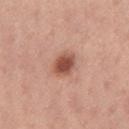The lesion was tiled from a total-body skin photograph and was not biopsied. Longest diameter approximately 3.5 mm. A 15 mm close-up extracted from a 3D total-body photography capture. From the leg. A female patient, about 25 years old. The lesion-visualizer software estimated a footprint of about 7 mm² and a shape eccentricity near 0.65. The analysis additionally found internal color variation of about 4 on a 0–10 scale and radial color variation of about 1. It also reported an automated nevus-likeness rating near 100 out of 100 and lesion-presence confidence of about 100/100. The tile uses white-light illumination.